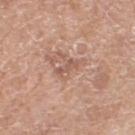Captured during whole-body skin photography for melanoma surveillance; the lesion was not biopsied. The lesion is on the left thigh. A female patient roughly 75 years of age. The total-body-photography lesion software estimated a border-irregularity index near 7/10 and radial color variation of about 0. The analysis additionally found a nevus-likeness score of about 0/100 and a detector confidence of about 95 out of 100 that the crop contains a lesion. Imaged with white-light lighting. Approximately 3 mm at its widest. This image is a 15 mm lesion crop taken from a total-body photograph.